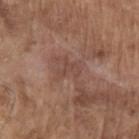biopsy_status: not biopsied; imaged during a skin examination
site: left upper arm
patient:
  sex: male
  age_approx: 80
lesion_size:
  long_diameter_mm_approx: 3.0
lighting: white-light
image:
  source: total-body photography crop
  field_of_view_mm: 15